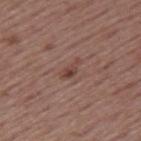Image and clinical context:
The lesion is located on the left upper arm. Longest diameter approximately 3 mm. This is a white-light tile. Cropped from a total-body skin-imaging series; the visible field is about 15 mm. The patient is a male aged approximately 55.